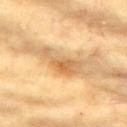Case summary:
* diameter · ≈5 mm
* subject · female, roughly 75 years of age
* lighting · cross-polarized illumination
* image · ~15 mm crop, total-body skin-cancer survey
* anatomic site · the left upper arm